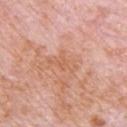Cropped from a total-body skin-imaging series; the visible field is about 15 mm.
Located on the chest.
About 4 mm across.
A male subject roughly 80 years of age.